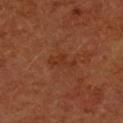Automated tile analysis of the lesion measured an average lesion color of about L≈33 a*≈25 b*≈32 (CIELAB), roughly 5 lightness units darker than nearby skin, and a normalized border contrast of about 5.5. And it measured a border-irregularity rating of about 5.5/10, internal color variation of about 0.5 on a 0–10 scale, and a peripheral color-asymmetry measure near 0. The software also gave an automated nevus-likeness rating near 0 out of 100 and lesion-presence confidence of about 100/100. A 15 mm crop from a total-body photograph taken for skin-cancer surveillance. On the left forearm. The tile uses cross-polarized illumination. The recorded lesion diameter is about 3.5 mm. The subject is a female roughly 65 years of age.